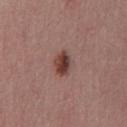Notes:
• follow-up · catalogued during a skin exam; not biopsied
• diameter · ≈3 mm
• imaging modality · total-body-photography crop, ~15 mm field of view
• location · the chest
• patient · male, approximately 40 years of age